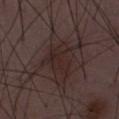{"biopsy_status": "not biopsied; imaged during a skin examination", "image": {"source": "total-body photography crop", "field_of_view_mm": 15}, "patient": {"sex": "male", "age_approx": 50}}